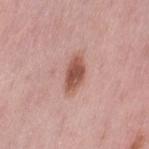follow-up: catalogued during a skin exam; not biopsied
site: the left thigh
patient: female, approximately 50 years of age
image source: ~15 mm crop, total-body skin-cancer survey